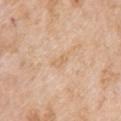notes: imaged on a skin check; not biopsied
acquisition: ~15 mm tile from a whole-body skin photo
TBP lesion metrics: a shape eccentricity near 0.9 and a shape-asymmetry score of about 0.4 (0 = symmetric); an average lesion color of about L≈67 a*≈17 b*≈36 (CIELAB), roughly 6 lightness units darker than nearby skin, and a lesion-to-skin contrast of about 5 (normalized; higher = more distinct); a border-irregularity index near 4/10, a within-lesion color-variation index near 0/10, and radial color variation of about 0
diameter: about 2.5 mm
subject: female, aged around 70
illumination: white-light illumination
body site: the arm